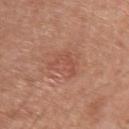notes: catalogued during a skin exam; not biopsied | lighting: white-light illumination | diameter: about 4 mm | patient: male, in their 70s | automated metrics: a footprint of about 6.5 mm², a shape eccentricity near 0.8, and two-axis asymmetry of about 0.5; an average lesion color of about L≈52 a*≈25 b*≈30 (CIELAB), a lesion–skin lightness drop of about 6, and a normalized lesion–skin contrast near 4.5; a detector confidence of about 100 out of 100 that the crop contains a lesion | body site: the left upper arm | image source: total-body-photography crop, ~15 mm field of view.